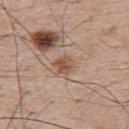Part of a total-body skin-imaging series; this lesion was reviewed on a skin check and was not flagged for biopsy. A male patient, aged 63 to 67. From the upper back. Cropped from a whole-body photographic skin survey; the tile spans about 15 mm. This is a white-light tile. Automated image analysis of the tile measured an area of roughly 4.5 mm² and a symmetry-axis asymmetry near 0.25. And it measured a classifier nevus-likeness of about 5/100 and a lesion-detection confidence of about 100/100. The recorded lesion diameter is about 2.5 mm.